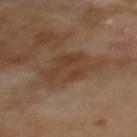No biopsy was performed on this lesion — it was imaged during a full skin examination and was not determined to be concerning.
This is a cross-polarized tile.
The patient is a male aged around 70.
A lesion tile, about 15 mm wide, cut from a 3D total-body photograph.
Located on the mid back.